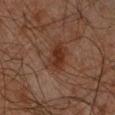biopsy status — total-body-photography surveillance lesion; no biopsy
site — the right forearm
image source — 15 mm crop, total-body photography
patient — male, in their mid-60s
image-analysis metrics — a within-lesion color-variation index near 3/10 and radial color variation of about 1
illumination — cross-polarized illumination